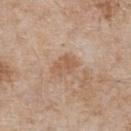Imaged during a routine full-body skin examination; the lesion was not biopsied and no histopathology is available. Located on the chest. Imaged with white-light lighting. A male subject, aged 63–67. A region of skin cropped from a whole-body photographic capture, roughly 15 mm wide.